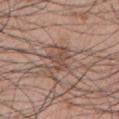Q: Is there a histopathology result?
A: imaged on a skin check; not biopsied
Q: How was this image acquired?
A: ~15 mm crop, total-body skin-cancer survey
Q: What is the anatomic site?
A: the chest
Q: How was the tile lit?
A: white-light illumination
Q: Who is the patient?
A: male, aged approximately 70
Q: How large is the lesion?
A: about 3.5 mm